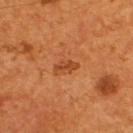This lesion was catalogued during total-body skin photography and was not selected for biopsy. The lesion's longest dimension is about 3 mm. The lesion-visualizer software estimated a lesion area of about 3 mm², a shape eccentricity near 0.9, and a symmetry-axis asymmetry near 0.2. It also reported a mean CIELAB color near L≈42 a*≈28 b*≈38 and a normalized border contrast of about 6.5. And it measured a border-irregularity index near 3/10 and peripheral color asymmetry of about 0. From the upper back. The tile uses cross-polarized illumination. The patient is a male aged approximately 55. This image is a 15 mm lesion crop taken from a total-body photograph.